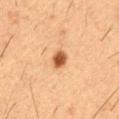  biopsy_status: not biopsied; imaged during a skin examination
  site: chest
  lesion_size:
    long_diameter_mm_approx: 2.5
  image:
    source: total-body photography crop
    field_of_view_mm: 15
  patient:
    sex: male
    age_approx: 55